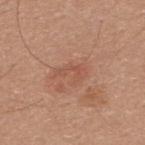Impression:
Part of a total-body skin-imaging series; this lesion was reviewed on a skin check and was not flagged for biopsy.
Clinical summary:
The lesion is on the upper back. A male patient in their 30s. Automated image analysis of the tile measured a lesion area of about 5 mm² and two-axis asymmetry of about 0.45. The software also gave an average lesion color of about L≈53 a*≈23 b*≈30 (CIELAB) and a lesion–skin lightness drop of about 6. The software also gave a border-irregularity index near 6.5/10, internal color variation of about 1 on a 0–10 scale, and peripheral color asymmetry of about 0.5. It also reported an automated nevus-likeness rating near 0 out of 100 and a lesion-detection confidence of about 100/100. Captured under white-light illumination. Approximately 3.5 mm at its widest. Cropped from a whole-body photographic skin survey; the tile spans about 15 mm.